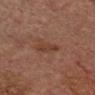Captured under cross-polarized illumination.
From the head or neck.
A male subject, approximately 75 years of age.
A 15 mm crop from a total-body photograph taken for skin-cancer surveillance.
Approximately 3 mm at its widest.